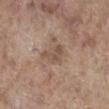{
  "patient": {
    "sex": "female",
    "age_approx": "85+"
  },
  "image": {
    "source": "total-body photography crop",
    "field_of_view_mm": 15
  },
  "lesion_size": {
    "long_diameter_mm_approx": 3.0
  },
  "site": "right lower leg"
}